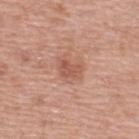biopsy status = imaged on a skin check; not biopsied | subject = male, about 55 years old | acquisition = ~15 mm tile from a whole-body skin photo | location = the upper back.